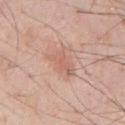This lesion was catalogued during total-body skin photography and was not selected for biopsy. Imaged with white-light lighting. Automated tile analysis of the lesion measured a mean CIELAB color near L≈62 a*≈22 b*≈28, roughly 7 lightness units darker than nearby skin, and a normalized border contrast of about 5. The software also gave a border-irregularity rating of about 4/10, internal color variation of about 2.5 on a 0–10 scale, and a peripheral color-asymmetry measure near 1. The analysis additionally found a nevus-likeness score of about 25/100 and a lesion-detection confidence of about 100/100. A male subject aged approximately 60. Cropped from a total-body skin-imaging series; the visible field is about 15 mm. The lesion is on the front of the torso.